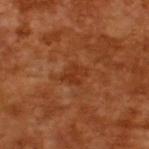Impression: Captured during whole-body skin photography for melanoma surveillance; the lesion was not biopsied. Context: The patient is a male about 65 years old. An algorithmic analysis of the crop reported an area of roughly 5 mm² and a symmetry-axis asymmetry near 0.25. The software also gave about 6 CIELAB-L* units darker than the surrounding skin and a normalized border contrast of about 5.5. The analysis additionally found an automated nevus-likeness rating near 0 out of 100. A 15 mm crop from a total-body photograph taken for skin-cancer surveillance. Captured under cross-polarized illumination.